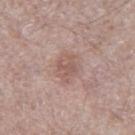This lesion was catalogued during total-body skin photography and was not selected for biopsy. The lesion-visualizer software estimated a footprint of about 5 mm², an outline eccentricity of about 0.6 (0 = round, 1 = elongated), and two-axis asymmetry of about 0.3. The software also gave an automated nevus-likeness rating near 0 out of 100 and a detector confidence of about 100 out of 100 that the crop contains a lesion. A lesion tile, about 15 mm wide, cut from a 3D total-body photograph. A male subject, aged approximately 70. On the leg. Approximately 3 mm at its widest.